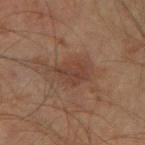Q: Is there a histopathology result?
A: total-body-photography surveillance lesion; no biopsy
Q: What is the imaging modality?
A: ~15 mm crop, total-body skin-cancer survey
Q: What is the lesion's diameter?
A: ≈4.5 mm
Q: Who is the patient?
A: male, approximately 60 years of age
Q: What is the anatomic site?
A: the left forearm
Q: What did automated image analysis measure?
A: a footprint of about 8.5 mm² and an outline eccentricity of about 0.8 (0 = round, 1 = elongated); an average lesion color of about L≈36 a*≈17 b*≈24 (CIELAB), about 7 CIELAB-L* units darker than the surrounding skin, and a normalized lesion–skin contrast near 6; a nevus-likeness score of about 5/100 and a detector confidence of about 100 out of 100 that the crop contains a lesion
Q: What lighting was used for the tile?
A: cross-polarized illumination